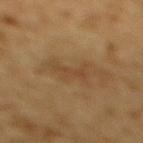Clinical summary:
Cropped from a total-body skin-imaging series; the visible field is about 15 mm. Captured under cross-polarized illumination. A male subject, approximately 85 years of age or older. Approximately 4 mm at its widest. On the mid back.